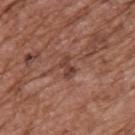| feature | finding |
|---|---|
| notes | no biopsy performed (imaged during a skin exam) |
| patient | male, aged 73–77 |
| image-analysis metrics | a footprint of about 3 mm², an outline eccentricity of about 0.85 (0 = round, 1 = elongated), and a shape-asymmetry score of about 0.35 (0 = symmetric); a lesion–skin lightness drop of about 9 and a lesion-to-skin contrast of about 7.5 (normalized; higher = more distinct); a border-irregularity rating of about 4/10, a color-variation rating of about 0/10, and radial color variation of about 0; an automated nevus-likeness rating near 0 out of 100 and lesion-presence confidence of about 95/100 |
| body site | the upper back |
| lesion diameter | about 2.5 mm |
| imaging modality | ~15 mm tile from a whole-body skin photo |
| lighting | white-light |